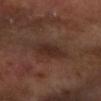Clinical impression: No biopsy was performed on this lesion — it was imaged during a full skin examination and was not determined to be concerning. Clinical summary: The tile uses cross-polarized illumination. A male subject aged 53–57. The lesion is located on the right forearm. The lesion's longest dimension is about 4 mm. This image is a 15 mm lesion crop taken from a total-body photograph.